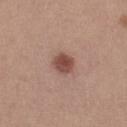The lesion was tiled from a total-body skin photograph and was not biopsied. A female subject aged approximately 20. This is a white-light tile. Automated image analysis of the tile measured an area of roughly 5.5 mm², an outline eccentricity of about 0.45 (0 = round, 1 = elongated), and a shape-asymmetry score of about 0.2 (0 = symmetric). It also reported an automated nevus-likeness rating near 95 out of 100 and a detector confidence of about 100 out of 100 that the crop contains a lesion. Cropped from a whole-body photographic skin survey; the tile spans about 15 mm. Measured at roughly 2.5 mm in maximum diameter. Located on the leg.